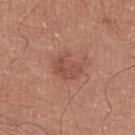{"biopsy_status": "not biopsied; imaged during a skin examination", "patient": {"sex": "male", "age_approx": 70}, "site": "right lower leg", "automated_metrics": {"cielab_L": 50, "cielab_a": 25, "cielab_b": 27, "vs_skin_darker_L": 8.0}, "image": {"source": "total-body photography crop", "field_of_view_mm": 15}, "lesion_size": {"long_diameter_mm_approx": 3.5}, "lighting": "white-light"}